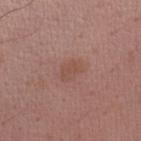  biopsy_status: not biopsied; imaged during a skin examination
  automated_metrics:
    vs_skin_darker_L: 6.0
    vs_skin_contrast_norm: 5.0
  site: right upper arm
  lighting: white-light
  patient:
    sex: male
    age_approx: 55
  lesion_size:
    long_diameter_mm_approx: 3.0
  image:
    source: total-body photography crop
    field_of_view_mm: 15